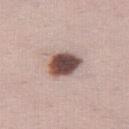Impression:
Part of a total-body skin-imaging series; this lesion was reviewed on a skin check and was not flagged for biopsy.
Image and clinical context:
The tile uses white-light illumination. The lesion's longest dimension is about 4 mm. Cropped from a total-body skin-imaging series; the visible field is about 15 mm. A female subject in their 40s. On the leg. An algorithmic analysis of the crop reported a lesion area of about 9.5 mm². The analysis additionally found a mean CIELAB color near L≈48 a*≈18 b*≈21, a lesion–skin lightness drop of about 22, and a normalized border contrast of about 14.5. And it measured internal color variation of about 6 on a 0–10 scale and a peripheral color-asymmetry measure near 1.5. The analysis additionally found a nevus-likeness score of about 85/100 and a lesion-detection confidence of about 100/100.